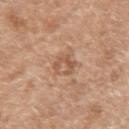biopsy status = imaged on a skin check; not biopsied
subject = male, roughly 60 years of age
acquisition = total-body-photography crop, ~15 mm field of view
anatomic site = the left upper arm